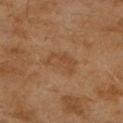{"biopsy_status": "not biopsied; imaged during a skin examination", "patient": {"sex": "female", "age_approx": 60}, "image": {"source": "total-body photography crop", "field_of_view_mm": 15}, "lesion_size": {"long_diameter_mm_approx": 4.0}, "site": "right upper arm", "automated_metrics": {"cielab_L": 42, "cielab_a": 18, "cielab_b": 32, "vs_skin_darker_L": 5.0, "vs_skin_contrast_norm": 5.0, "color_variation_0_10": 2.5, "peripheral_color_asymmetry": 1.0}}